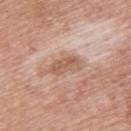biopsy_status: not biopsied; imaged during a skin examination
patient:
  sex: female
  age_approx: 60
site: upper back
lesion_size:
  long_diameter_mm_approx: 4.0
automated_metrics:
  cielab_L: 58
  cielab_a: 20
  cielab_b: 30
  vs_skin_darker_L: 9.0
  vs_skin_contrast_norm: 6.5
image:
  source: total-body photography crop
  field_of_view_mm: 15